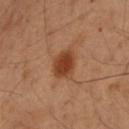biopsy status=imaged on a skin check; not biopsied | location=the left arm | image-analysis metrics=a lesion color around L≈34 a*≈21 b*≈29 in CIELAB, roughly 10 lightness units darker than nearby skin, and a normalized lesion–skin contrast near 9; a border-irregularity index near 1.5/10 and a within-lesion color-variation index near 2.5/10; an automated nevus-likeness rating near 100 out of 100 and lesion-presence confidence of about 100/100 | imaging modality=15 mm crop, total-body photography | subject=female, roughly 55 years of age | size=~3 mm (longest diameter).